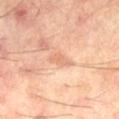The lesion was tiled from a total-body skin photograph and was not biopsied.
Automated tile analysis of the lesion measured a lesion color around L≈65 a*≈22 b*≈33 in CIELAB and roughly 8 lightness units darker than nearby skin.
Longest diameter approximately 3 mm.
A male patient, aged approximately 60.
A region of skin cropped from a whole-body photographic capture, roughly 15 mm wide.
Located on the right thigh.